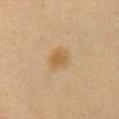Findings:
- tile lighting · cross-polarized illumination
- subject · female, aged 38–42
- site · the chest
- image-analysis metrics · a lesion color around L≈47 a*≈14 b*≈33 in CIELAB, a lesion–skin lightness drop of about 7, and a lesion-to-skin contrast of about 7 (normalized; higher = more distinct); border irregularity of about 1.5 on a 0–10 scale, a color-variation rating of about 2/10, and peripheral color asymmetry of about 0.5; a nevus-likeness score of about 95/100 and a detector confidence of about 100 out of 100 that the crop contains a lesion
- acquisition · 15 mm crop, total-body photography
- lesion size · ≈2.5 mm